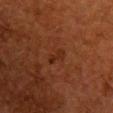No biopsy was performed on this lesion — it was imaged during a full skin examination and was not determined to be concerning. About 2.5 mm across. Cropped from a total-body skin-imaging series; the visible field is about 15 mm. This is a cross-polarized tile. A female patient aged 48 to 52. On the chest.